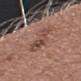  biopsy_status: not biopsied; imaged during a skin examination
  lesion_size:
    long_diameter_mm_approx: 5.0
  automated_metrics:
    area_mm2_approx: 8.0
    eccentricity: 0.9
    shape_asymmetry: 0.4
    cielab_L: 47
    cielab_a: 21
    cielab_b: 24
    vs_skin_darker_L: 9.0
    vs_skin_contrast_norm: 6.5
    border_irregularity_0_10: 6.0
    color_variation_0_10: 4.5
    nevus_likeness_0_100: 0
    lesion_detection_confidence_0_100: 100
  patient:
    sex: female
    age_approx: 50
  site: left forearm
  image:
    source: total-body photography crop
    field_of_view_mm: 15